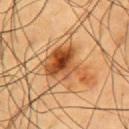Q: Was a biopsy performed?
A: imaged on a skin check; not biopsied
Q: What is the anatomic site?
A: the chest
Q: What kind of image is this?
A: ~15 mm tile from a whole-body skin photo
Q: What lighting was used for the tile?
A: cross-polarized illumination
Q: Who is the patient?
A: male, about 60 years old
Q: What is the lesion's diameter?
A: ~6 mm (longest diameter)